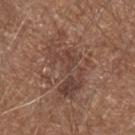Case summary:
– follow-up — catalogued during a skin exam; not biopsied
– subject — male, about 65 years old
– image — 15 mm crop, total-body photography
– tile lighting — white-light
– body site — the left forearm
– lesion diameter — ~8 mm (longest diameter)